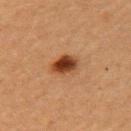lesion diameter: about 3.5 mm
subject: female, in their 60s
image source: total-body-photography crop, ~15 mm field of view
site: the left upper arm
automated metrics: a border-irregularity rating of about 1.5/10 and internal color variation of about 5.5 on a 0–10 scale; a nevus-likeness score of about 100/100 and lesion-presence confidence of about 100/100